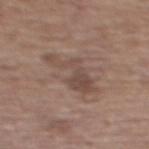Assessment:
Recorded during total-body skin imaging; not selected for excision or biopsy.
Context:
A 15 mm crop from a total-body photograph taken for skin-cancer surveillance. On the upper back. The subject is a female about 65 years old.